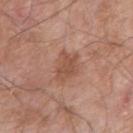<case>
  <biopsy_status>not biopsied; imaged during a skin examination</biopsy_status>
  <site>right upper arm</site>
  <patient>
    <sex>male</sex>
    <age_approx>75</age_approx>
  </patient>
  <lighting>white-light</lighting>
  <automated_metrics>
    <border_irregularity_0_10>3.5</border_irregularity_0_10>
    <color_variation_0_10>2.5</color_variation_0_10>
  </automated_metrics>
  <image>
    <source>total-body photography crop</source>
    <field_of_view_mm>15</field_of_view_mm>
  </image>
  <lesion_size>
    <long_diameter_mm_approx>3.5</long_diameter_mm_approx>
  </lesion_size>
</case>